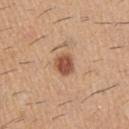Findings:
* notes · imaged on a skin check; not biopsied
* subject · male, roughly 60 years of age
* site · the right upper arm
* size · ≈3 mm
* image · total-body-photography crop, ~15 mm field of view
* lighting · white-light